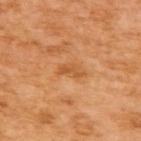A lesion tile, about 15 mm wide, cut from a 3D total-body photograph. From the upper back. The subject is a female approximately 55 years of age.